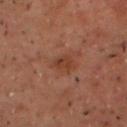Impression: Captured during whole-body skin photography for melanoma surveillance; the lesion was not biopsied. Acquisition and patient details: Located on the head or neck. The patient is a male aged approximately 50. This is a cross-polarized tile. An algorithmic analysis of the crop reported a footprint of about 4 mm², an eccentricity of roughly 0.7, and two-axis asymmetry of about 0.25. The software also gave an automated nevus-likeness rating near 0 out of 100 and a lesion-detection confidence of about 100/100. The recorded lesion diameter is about 2.5 mm. A 15 mm close-up extracted from a 3D total-body photography capture.